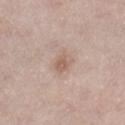Imaged during a routine full-body skin examination; the lesion was not biopsied and no histopathology is available. The total-body-photography lesion software estimated an average lesion color of about L≈60 a*≈17 b*≈26 (CIELAB), a lesion–skin lightness drop of about 8, and a normalized border contrast of about 6. The software also gave a nevus-likeness score of about 10/100 and a detector confidence of about 100 out of 100 that the crop contains a lesion. The subject is a female aged around 40. Imaged with white-light lighting. On the right lower leg. A 15 mm close-up tile from a total-body photography series done for melanoma screening. Approximately 2.5 mm at its widest.